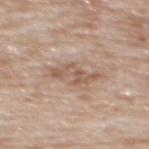Q: Is there a histopathology result?
A: no biopsy performed (imaged during a skin exam)
Q: What is the anatomic site?
A: the mid back
Q: Who is the patient?
A: male, in their 80s
Q: Illumination type?
A: white-light
Q: How was this image acquired?
A: 15 mm crop, total-body photography
Q: How large is the lesion?
A: about 5 mm